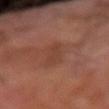Q: Was this lesion biopsied?
A: total-body-photography surveillance lesion; no biopsy
Q: What are the patient's age and sex?
A: male, in their 70s
Q: What is the anatomic site?
A: the right forearm
Q: Automated lesion metrics?
A: a lesion color around L≈40 a*≈23 b*≈27 in CIELAB, roughly 5 lightness units darker than nearby skin, and a normalized lesion–skin contrast near 5
Q: How large is the lesion?
A: ≈4 mm
Q: What kind of image is this?
A: ~15 mm tile from a whole-body skin photo